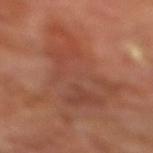| feature | finding |
|---|---|
| biopsy status | total-body-photography surveillance lesion; no biopsy |
| automated lesion analysis | a lesion-detection confidence of about 90/100 |
| anatomic site | the right lower leg |
| acquisition | total-body-photography crop, ~15 mm field of view |
| lesion diameter | about 11.5 mm |
| patient | male, aged around 70 |
| lighting | cross-polarized |